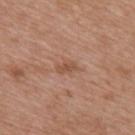No biopsy was performed on this lesion — it was imaged during a full skin examination and was not determined to be concerning. From the back. This is a white-light tile. A male patient, aged 48 to 52. Cropped from a whole-body photographic skin survey; the tile spans about 15 mm.